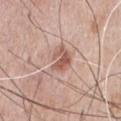<lesion>
  <biopsy_status>not biopsied; imaged during a skin examination</biopsy_status>
  <automated_metrics>
    <cielab_L>59</cielab_L>
    <cielab_a>21</cielab_a>
    <cielab_b>26</cielab_b>
    <vs_skin_darker_L>10.0</vs_skin_darker_L>
    <vs_skin_contrast_norm>6.5</vs_skin_contrast_norm>
    <nevus_likeness_0_100>5</nevus_likeness_0_100>
    <lesion_detection_confidence_0_100>100</lesion_detection_confidence_0_100>
  </automated_metrics>
  <lesion_size>
    <long_diameter_mm_approx>4.5</long_diameter_mm_approx>
  </lesion_size>
  <patient>
    <sex>male</sex>
    <age_approx>80</age_approx>
  </patient>
  <image>
    <source>total-body photography crop</source>
    <field_of_view_mm>15</field_of_view_mm>
  </image>
  <lighting>white-light</lighting>
  <site>chest</site>
</lesion>